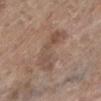No biopsy was performed on this lesion — it was imaged during a full skin examination and was not determined to be concerning.
From the left lower leg.
This is a white-light tile.
The patient is a female in their mid- to late 80s or older.
A lesion tile, about 15 mm wide, cut from a 3D total-body photograph.
Longest diameter approximately 5.5 mm.